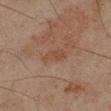No biopsy was performed on this lesion — it was imaged during a full skin examination and was not determined to be concerning. A 15 mm crop from a total-body photograph taken for skin-cancer surveillance. The lesion's longest dimension is about 3 mm. Imaged with cross-polarized lighting. From the right lower leg. A male patient, aged 43 to 47.